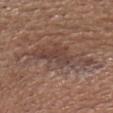No biopsy was performed on this lesion — it was imaged during a full skin examination and was not determined to be concerning. A male subject aged 73–77. The lesion is located on the head or neck. A roughly 15 mm field-of-view crop from a total-body skin photograph. Longest diameter approximately 10 mm.